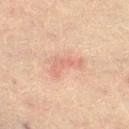Captured during whole-body skin photography for melanoma surveillance; the lesion was not biopsied. About 3.5 mm across. This is a cross-polarized tile. A male subject approximately 60 years of age. The lesion is on the left thigh. A close-up tile cropped from a whole-body skin photograph, about 15 mm across.